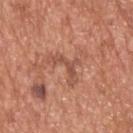Assessment:
Recorded during total-body skin imaging; not selected for excision or biopsy.
Acquisition and patient details:
The lesion is located on the upper back. Imaged with white-light lighting. Longest diameter approximately 4 mm. A male subject, aged approximately 65. Cropped from a total-body skin-imaging series; the visible field is about 15 mm.